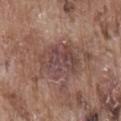Clinical impression:
Recorded during total-body skin imaging; not selected for excision or biopsy.
Background:
This is a white-light tile. A male patient, aged 73–77. A region of skin cropped from a whole-body photographic capture, roughly 15 mm wide. The lesion-visualizer software estimated a color-variation rating of about 4.5/10 and peripheral color asymmetry of about 1.5. The analysis additionally found an automated nevus-likeness rating near 0 out of 100. The lesion is located on the lower back.